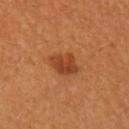biopsy_status: not biopsied; imaged during a skin examination
automated_metrics:
  border_irregularity_0_10: 2.0
  color_variation_0_10: 3.0
  peripheral_color_asymmetry: 1.0
site: left upper arm
image:
  source: total-body photography crop
  field_of_view_mm: 15
lesion_size:
  long_diameter_mm_approx: 3.0
patient:
  sex: female
  age_approx: 50